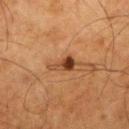<tbp_lesion>
  <biopsy_status>not biopsied; imaged during a skin examination</biopsy_status>
  <lesion_size>
    <long_diameter_mm_approx>3.5</long_diameter_mm_approx>
  </lesion_size>
  <site>right thigh</site>
  <patient>
    <sex>male</sex>
    <age_approx>80</age_approx>
  </patient>
  <automated_metrics>
    <eccentricity>0.9</eccentricity>
    <shape_asymmetry>0.45</shape_asymmetry>
    <border_irregularity_0_10>5.0</border_irregularity_0_10>
    <color_variation_0_10>2.5</color_variation_0_10>
    <peripheral_color_asymmetry>0.5</peripheral_color_asymmetry>
  </automated_metrics>
  <image>
    <source>total-body photography crop</source>
    <field_of_view_mm>15</field_of_view_mm>
  </image>
</tbp_lesion>